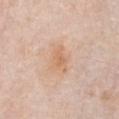Part of a total-body skin-imaging series; this lesion was reviewed on a skin check and was not flagged for biopsy. The lesion is on the chest. Imaged with white-light lighting. A male subject about 75 years old. A 15 mm close-up extracted from a 3D total-body photography capture.